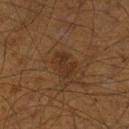{
  "biopsy_status": "not biopsied; imaged during a skin examination",
  "automated_metrics": {
    "area_mm2_approx": 5.5,
    "eccentricity": 0.65,
    "shape_asymmetry": 0.3,
    "color_variation_0_10": 1.5,
    "peripheral_color_asymmetry": 0.5,
    "nevus_likeness_0_100": 35
  },
  "lesion_size": {
    "long_diameter_mm_approx": 3.0
  },
  "image": {
    "source": "total-body photography crop",
    "field_of_view_mm": 15
  },
  "lighting": "cross-polarized",
  "patient": {
    "sex": "male",
    "age_approx": 60
  },
  "site": "leg"
}